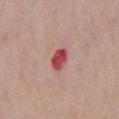The lesion was photographed on a routine skin check and not biopsied; there is no pathology result. A male patient aged 58 to 62. The lesion is located on the chest. Captured under white-light illumination. This image is a 15 mm lesion crop taken from a total-body photograph. Measured at roughly 2.5 mm in maximum diameter. Automated image analysis of the tile measured an area of roughly 4.5 mm², a shape eccentricity near 0.45, and a shape-asymmetry score of about 0.2 (0 = symmetric). The analysis additionally found a lesion-to-skin contrast of about 10.5 (normalized; higher = more distinct). The analysis additionally found a border-irregularity index near 2/10. The software also gave a classifier nevus-likeness of about 0/100 and lesion-presence confidence of about 100/100.